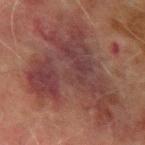Assessment:
Recorded during total-body skin imaging; not selected for excision or biopsy.
Image and clinical context:
The lesion is on the left forearm. Approximately 11 mm at its widest. Cropped from a total-body skin-imaging series; the visible field is about 15 mm. The total-body-photography lesion software estimated border irregularity of about 9.5 on a 0–10 scale, a color-variation rating of about 5/10, and a peripheral color-asymmetry measure near 1.5. Imaged with cross-polarized lighting. A male patient, aged 68 to 72.